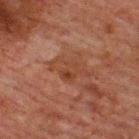Captured during whole-body skin photography for melanoma surveillance; the lesion was not biopsied. Longest diameter approximately 5 mm. Automated tile analysis of the lesion measured a lesion color around L≈32 a*≈19 b*≈25 in CIELAB, about 5 CIELAB-L* units darker than the surrounding skin, and a lesion-to-skin contrast of about 5.5 (normalized; higher = more distinct). The software also gave a border-irregularity rating of about 4.5/10 and internal color variation of about 3.5 on a 0–10 scale. It also reported a nevus-likeness score of about 0/100 and lesion-presence confidence of about 100/100. From the upper back. A 15 mm close-up extracted from a 3D total-body photography capture. A male patient, in their 60s. Imaged with cross-polarized lighting.